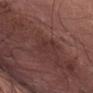- notes: imaged on a skin check; not biopsied
- location: the abdomen
- image source: 15 mm crop, total-body photography
- lesion size: ≈3 mm
- subject: male, aged around 75